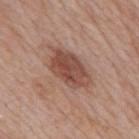Recorded during total-body skin imaging; not selected for excision or biopsy.